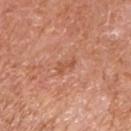The lesion was tiled from a total-body skin photograph and was not biopsied. Located on the left upper arm. A close-up tile cropped from a whole-body skin photograph, about 15 mm across. A male subject aged 63–67.